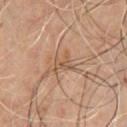workup: no biopsy performed (imaged during a skin exam) | site: the chest | patient: male, in their mid- to late 40s | acquisition: total-body-photography crop, ~15 mm field of view | size: ~3 mm (longest diameter).